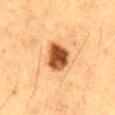Recorded during total-body skin imaging; not selected for excision or biopsy.
Automated tile analysis of the lesion measured a mean CIELAB color near L≈47 a*≈25 b*≈38, a lesion–skin lightness drop of about 20, and a lesion-to-skin contrast of about 13 (normalized; higher = more distinct). The software also gave a border-irregularity rating of about 2/10, a within-lesion color-variation index near 6/10, and radial color variation of about 2.
About 4 mm across.
A 15 mm close-up extracted from a 3D total-body photography capture.
On the front of the torso.
A male patient approximately 85 years of age or older.
Imaged with cross-polarized lighting.